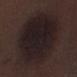follow-up = no biopsy performed (imaged during a skin exam)
tile lighting = white-light illumination
subject = male, in their 70s
imaging modality = ~15 mm tile from a whole-body skin photo
TBP lesion metrics = an area of roughly 65 mm² and an eccentricity of roughly 0.6; a mean CIELAB color near L≈17 a*≈10 b*≈11, roughly 7 lightness units darker than nearby skin, and a normalized border contrast of about 11; border irregularity of about 1.5 on a 0–10 scale and a within-lesion color-variation index near 4/10; a classifier nevus-likeness of about 5/100 and a detector confidence of about 100 out of 100 that the crop contains a lesion
anatomic site = the left lower leg
lesion diameter = about 10.5 mm